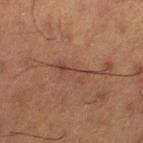- follow-up: catalogued during a skin exam; not biopsied
- lesion diameter: ≈4 mm
- body site: the leg
- tile lighting: cross-polarized
- imaging modality: 15 mm crop, total-body photography
- subject: male, approximately 60 years of age